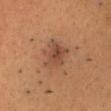biopsy_status: not biopsied; imaged during a skin examination
image:
  source: total-body photography crop
  field_of_view_mm: 15
site: head or neck
patient:
  sex: male
  age_approx: 40